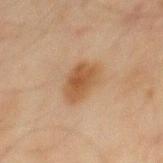follow-up = total-body-photography surveillance lesion; no biopsy
acquisition = total-body-photography crop, ~15 mm field of view
TBP lesion metrics = a mean CIELAB color near L≈43 a*≈17 b*≈31, about 9 CIELAB-L* units darker than the surrounding skin, and a normalized border contrast of about 8; a border-irregularity index near 2.5/10 and radial color variation of about 1
lighting = cross-polarized
location = the back
lesion size = ~4 mm (longest diameter)
subject = male, aged 43 to 47